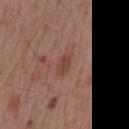<record>
<biopsy_status>not biopsied; imaged during a skin examination</biopsy_status>
<lesion_size>
  <long_diameter_mm_approx>2.5</long_diameter_mm_approx>
</lesion_size>
<site>mid back</site>
<image>
  <source>total-body photography crop</source>
  <field_of_view_mm>15</field_of_view_mm>
</image>
<lighting>white-light</lighting>
<patient>
  <sex>male</sex>
  <age_approx>55</age_approx>
</patient>
<automated_metrics>
  <cielab_L>44</cielab_L>
  <cielab_a>21</cielab_a>
  <cielab_b>26</cielab_b>
  <vs_skin_darker_L>8.0</vs_skin_darker_L>
  <vs_skin_contrast_norm>6.5</vs_skin_contrast_norm>
  <color_variation_0_10>2.0</color_variation_0_10>
  <peripheral_color_asymmetry>1.0</peripheral_color_asymmetry>
  <lesion_detection_confidence_0_100>100</lesion_detection_confidence_0_100>
</automated_metrics>
</record>